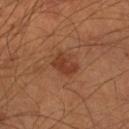biopsy status: total-body-photography surveillance lesion; no biopsy
site: the right forearm
imaging modality: ~15 mm tile from a whole-body skin photo
automated lesion analysis: about 8 CIELAB-L* units darker than the surrounding skin and a lesion-to-skin contrast of about 7 (normalized; higher = more distinct); border irregularity of about 2.5 on a 0–10 scale and a within-lesion color-variation index near 2/10; a nevus-likeness score of about 60/100
diameter: ≈3 mm
subject: male, aged approximately 65
illumination: cross-polarized illumination